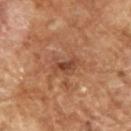Impression:
Captured during whole-body skin photography for melanoma surveillance; the lesion was not biopsied.
Context:
This is a cross-polarized tile. A 15 mm close-up extracted from a 3D total-body photography capture. Measured at roughly 3 mm in maximum diameter. Automated tile analysis of the lesion measured a footprint of about 4 mm², an eccentricity of roughly 0.75, and a shape-asymmetry score of about 0.35 (0 = symmetric). And it measured border irregularity of about 4.5 on a 0–10 scale and radial color variation of about 0.5. A male patient in their mid-60s.